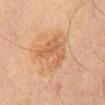  biopsy_status: not biopsied; imaged during a skin examination
  automated_metrics:
    eccentricity: 0.6
    shape_asymmetry: 0.4
    cielab_L: 50
    cielab_a: 19
    cielab_b: 31
    vs_skin_contrast_norm: 5.5
    nevus_likeness_0_100: 25
    lesion_detection_confidence_0_100: 100
  lighting: cross-polarized
  site: abdomen
  lesion_size:
    long_diameter_mm_approx: 5.5
  image:
    source: total-body photography crop
    field_of_view_mm: 15
  patient:
    sex: male
    age_approx: 70